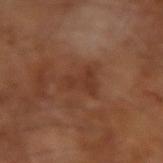Notes:
• image source — ~15 mm tile from a whole-body skin photo
• lighting — cross-polarized illumination
• automated lesion analysis — a footprint of about 7 mm², a shape eccentricity near 0.65, and a symmetry-axis asymmetry near 0.5; a mean CIELAB color near L≈34 a*≈21 b*≈29, roughly 6 lightness units darker than nearby skin, and a normalized lesion–skin contrast near 5.5; a color-variation rating of about 1.5/10 and a peripheral color-asymmetry measure near 0.5
• lesion size — ~3.5 mm (longest diameter)
• subject — male, about 65 years old